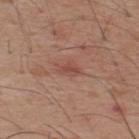Recorded during total-body skin imaging; not selected for excision or biopsy.
Approximately 2.5 mm at its widest.
The tile uses white-light illumination.
The lesion is on the upper back.
A 15 mm close-up tile from a total-body photography series done for melanoma screening.
The patient is a male aged 38–42.